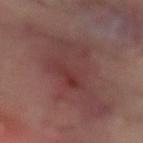{"site": "front of the torso", "automated_metrics": {"eccentricity": 0.9, "shape_asymmetry": 0.45, "cielab_L": 36, "cielab_a": 20, "cielab_b": 18, "vs_skin_contrast_norm": 6.5, "border_irregularity_0_10": 7.5, "color_variation_0_10": 4.5, "peripheral_color_asymmetry": 1.5}, "image": {"source": "total-body photography crop", "field_of_view_mm": 15}, "patient": {"sex": "male", "age_approx": 60}, "lesion_size": {"long_diameter_mm_approx": 12.5}}